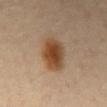<record>
<biopsy_status>not biopsied; imaged during a skin examination</biopsy_status>
<automated_metrics>
  <cielab_L>45</cielab_L>
  <cielab_a>19</cielab_a>
  <cielab_b>33</cielab_b>
  <vs_skin_darker_L>12.0</vs_skin_darker_L>
  <nevus_likeness_0_100>100</nevus_likeness_0_100>
</automated_metrics>
<lesion_size>
  <long_diameter_mm_approx>4.5</long_diameter_mm_approx>
</lesion_size>
<lighting>cross-polarized</lighting>
<patient>
  <sex>female</sex>
  <age_approx>65</age_approx>
</patient>
<image>
  <source>total-body photography crop</source>
  <field_of_view_mm>15</field_of_view_mm>
</image>
<site>back</site>
</record>